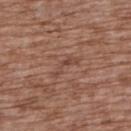* notes · imaged on a skin check; not biopsied